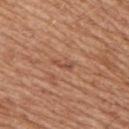Q: Was a biopsy performed?
A: imaged on a skin check; not biopsied
Q: How was the tile lit?
A: white-light
Q: Patient demographics?
A: male, aged 63–67
Q: How was this image acquired?
A: ~15 mm tile from a whole-body skin photo
Q: What did automated image analysis measure?
A: a footprint of about 2 mm² and a shape-asymmetry score of about 0.35 (0 = symmetric); border irregularity of about 4.5 on a 0–10 scale and peripheral color asymmetry of about 0; a classifier nevus-likeness of about 0/100 and a detector confidence of about 95 out of 100 that the crop contains a lesion
Q: Where on the body is the lesion?
A: the right upper arm
Q: What is the lesion's diameter?
A: ≈2.5 mm